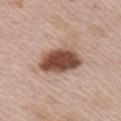workup: imaged on a skin check; not biopsied | illumination: white-light | image: ~15 mm crop, total-body skin-cancer survey | automated metrics: a mean CIELAB color near L≈50 a*≈20 b*≈26, roughly 18 lightness units darker than nearby skin, and a normalized lesion–skin contrast near 12; a within-lesion color-variation index near 6.5/10 and a peripheral color-asymmetry measure near 2; an automated nevus-likeness rating near 100 out of 100 and a detector confidence of about 100 out of 100 that the crop contains a lesion | subject: male, aged around 65 | location: the chest.